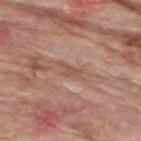notes = catalogued during a skin exam; not biopsied | location = the upper back | lighting = white-light illumination | patient = male, aged 58 to 62 | lesion diameter = ~3 mm (longest diameter) | image source = total-body-photography crop, ~15 mm field of view.